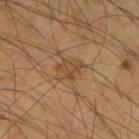workup — catalogued during a skin exam; not biopsied
tile lighting — cross-polarized
imaging modality — 15 mm crop, total-body photography
location — the right upper arm
size — about 3.5 mm
image-analysis metrics — a normalized lesion–skin contrast near 6; border irregularity of about 3.5 on a 0–10 scale, a within-lesion color-variation index near 3.5/10, and radial color variation of about 1.5
subject — male, approximately 65 years of age